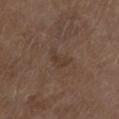This lesion was catalogued during total-body skin photography and was not selected for biopsy.
A male subject in their mid- to late 70s.
A 15 mm close-up tile from a total-body photography series done for melanoma screening.
The lesion is on the leg.